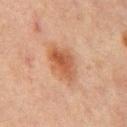{"image": {"source": "total-body photography crop", "field_of_view_mm": 15}, "automated_metrics": {"area_mm2_approx": 11.0, "eccentricity": 0.8, "shape_asymmetry": 0.2}, "lighting": "cross-polarized", "site": "mid back", "patient": {"sex": "male", "age_approx": 65}, "lesion_size": {"long_diameter_mm_approx": 5.0}}